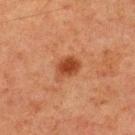Part of a total-body skin-imaging series; this lesion was reviewed on a skin check and was not flagged for biopsy. Automated image analysis of the tile measured a lesion color around L≈37 a*≈23 b*≈31 in CIELAB, about 9 CIELAB-L* units darker than the surrounding skin, and a lesion-to-skin contrast of about 8.5 (normalized; higher = more distinct). And it measured a classifier nevus-likeness of about 95/100. Longest diameter approximately 3.5 mm. Located on the back. Cropped from a whole-body photographic skin survey; the tile spans about 15 mm. A male subject, about 60 years old. Captured under cross-polarized illumination.